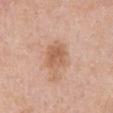Clinical impression:
No biopsy was performed on this lesion — it was imaged during a full skin examination and was not determined to be concerning.
Image and clinical context:
A male patient, in their 70s. A 15 mm close-up extracted from a 3D total-body photography capture. The lesion is on the abdomen.